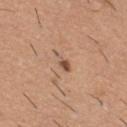Case summary:
• biopsy status — catalogued during a skin exam; not biopsied
• anatomic site — the mid back
• subject — male, in their 60s
• acquisition — 15 mm crop, total-body photography